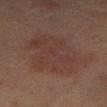{
  "biopsy_status": "not biopsied; imaged during a skin examination",
  "lesion_size": {
    "long_diameter_mm_approx": 8.5
  },
  "site": "right lower leg",
  "automated_metrics": {
    "border_irregularity_0_10": 3.5,
    "color_variation_0_10": 3.0,
    "nevus_likeness_0_100": 0
  },
  "image": {
    "source": "total-body photography crop",
    "field_of_view_mm": 15
  },
  "patient": {
    "sex": "female",
    "age_approx": 70
  }
}